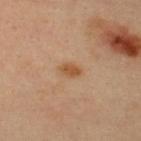Clinical impression: The lesion was tiled from a total-body skin photograph and was not biopsied. Clinical summary: Located on the back. This is a cross-polarized tile. A lesion tile, about 15 mm wide, cut from a 3D total-body photograph. A male patient, aged approximately 35. The total-body-photography lesion software estimated a shape eccentricity near 0.8. The analysis additionally found about 9 CIELAB-L* units darker than the surrounding skin and a normalized lesion–skin contrast near 7.5.